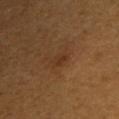image = ~15 mm crop, total-body skin-cancer survey
patient = male, approximately 50 years of age
site = the right upper arm
size = ~2 mm (longest diameter)
tile lighting = cross-polarized illumination